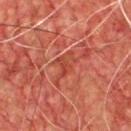No biopsy was performed on this lesion — it was imaged during a full skin examination and was not determined to be concerning.
A male subject aged approximately 65.
A 15 mm crop from a total-body photograph taken for skin-cancer surveillance.
The lesion is located on the chest.